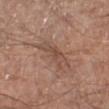The lesion was tiled from a total-body skin photograph and was not biopsied.
A 15 mm close-up tile from a total-body photography series done for melanoma screening.
The tile uses white-light illumination.
The patient is a male about 65 years old.
Located on the left lower leg.
The total-body-photography lesion software estimated a footprint of about 7.5 mm² and a shape-asymmetry score of about 0.5 (0 = symmetric). The analysis additionally found a nevus-likeness score of about 0/100 and a lesion-detection confidence of about 85/100.
Measured at roughly 5 mm in maximum diameter.